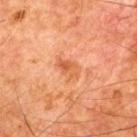The lesion was tiled from a total-body skin photograph and was not biopsied.
The recorded lesion diameter is about 3 mm.
Captured under cross-polarized illumination.
A lesion tile, about 15 mm wide, cut from a 3D total-body photograph.
The lesion is located on the upper back.
The subject is a male approximately 65 years of age.